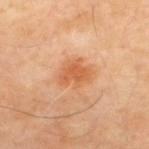Part of a total-body skin-imaging series; this lesion was reviewed on a skin check and was not flagged for biopsy. Automated tile analysis of the lesion measured a lesion-detection confidence of about 100/100. Measured at roughly 3.5 mm in maximum diameter. From the upper back. A 15 mm close-up tile from a total-body photography series done for melanoma screening. A male subject approximately 65 years of age. This is a cross-polarized tile.